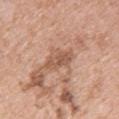Notes:
* biopsy status: no biopsy performed (imaged during a skin exam)
* lesion diameter: ~4.5 mm (longest diameter)
* anatomic site: the upper back
* patient: female, roughly 40 years of age
* image: ~15 mm crop, total-body skin-cancer survey